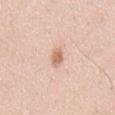Imaged during a routine full-body skin examination; the lesion was not biopsied and no histopathology is available.
Captured under white-light illumination.
Longest diameter approximately 2.5 mm.
A 15 mm close-up extracted from a 3D total-body photography capture.
From the mid back.
A male subject in their mid- to late 30s.